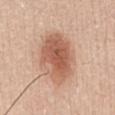Part of a total-body skin-imaging series; this lesion was reviewed on a skin check and was not flagged for biopsy. From the abdomen. The recorded lesion diameter is about 7 mm. This image is a 15 mm lesion crop taken from a total-body photograph. Captured under white-light illumination. The patient is a male roughly 35 years of age.